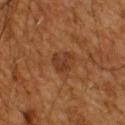Q: Was this lesion biopsied?
A: total-body-photography surveillance lesion; no biopsy
Q: How was this image acquired?
A: ~15 mm tile from a whole-body skin photo
Q: Lesion location?
A: the upper back
Q: Who is the patient?
A: male, aged 58–62